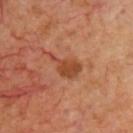Recorded during total-body skin imaging; not selected for excision or biopsy. A close-up tile cropped from a whole-body skin photograph, about 15 mm across. The subject is a male aged 68–72. From the chest. Measured at roughly 4.5 mm in maximum diameter. This is a cross-polarized tile.